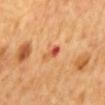The lesion was photographed on a routine skin check and not biopsied; there is no pathology result. A region of skin cropped from a whole-body photographic capture, roughly 15 mm wide. Located on the mid back. Measured at roughly 3 mm in maximum diameter. This is a cross-polarized tile. An algorithmic analysis of the crop reported an average lesion color of about L≈58 a*≈33 b*≈40 (CIELAB), roughly 12 lightness units darker than nearby skin, and a lesion-to-skin contrast of about 7.5 (normalized; higher = more distinct). It also reported a border-irregularity rating of about 3.5/10, a color-variation rating of about 3.5/10, and peripheral color asymmetry of about 0. A male subject, roughly 65 years of age.